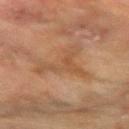Impression:
Imaged during a routine full-body skin examination; the lesion was not biopsied and no histopathology is available.
Image and clinical context:
A female patient aged around 55. A region of skin cropped from a whole-body photographic capture, roughly 15 mm wide. On the front of the torso. Captured under cross-polarized illumination.